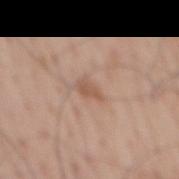A lesion tile, about 15 mm wide, cut from a 3D total-body photograph. On the back. A male subject aged approximately 70.